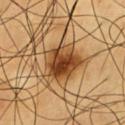follow-up: no biopsy performed (imaged during a skin exam)
lighting: cross-polarized illumination
image source: 15 mm crop, total-body photography
image-analysis metrics: an area of roughly 15 mm², a shape eccentricity near 0.4, and a shape-asymmetry score of about 0.2 (0 = symmetric); an average lesion color of about L≈45 a*≈22 b*≈39 (CIELAB), about 16 CIELAB-L* units darker than the surrounding skin, and a normalized border contrast of about 11.5; border irregularity of about 2 on a 0–10 scale, internal color variation of about 9.5 on a 0–10 scale, and radial color variation of about 3.5
lesion diameter: ≈4.5 mm
subject: male, in their 60s
site: the chest